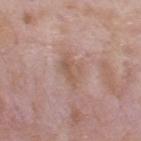Clinical impression: Captured during whole-body skin photography for melanoma surveillance; the lesion was not biopsied. Background: The patient is a male aged 53–57. The total-body-photography lesion software estimated a footprint of about 7 mm², an eccentricity of roughly 0.75, and two-axis asymmetry of about 0.3. And it measured a lesion color around L≈56 a*≈18 b*≈26 in CIELAB and a lesion-to-skin contrast of about 6 (normalized; higher = more distinct). And it measured a classifier nevus-likeness of about 0/100 and a lesion-detection confidence of about 100/100. A lesion tile, about 15 mm wide, cut from a 3D total-body photograph. Longest diameter approximately 4 mm. The lesion is on the back. Captured under white-light illumination.